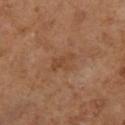Imaged during a routine full-body skin examination; the lesion was not biopsied and no histopathology is available.
Captured under cross-polarized illumination.
A female patient in their mid-60s.
From the left lower leg.
A 15 mm close-up tile from a total-body photography series done for melanoma screening.
Approximately 2.5 mm at its widest.